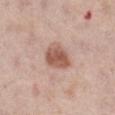No biopsy was performed on this lesion — it was imaged during a full skin examination and was not determined to be concerning. A lesion tile, about 15 mm wide, cut from a 3D total-body photograph. The lesion-visualizer software estimated an area of roughly 9 mm² and an eccentricity of roughly 0.55. The software also gave an average lesion color of about L≈56 a*≈21 b*≈27 (CIELAB), a lesion–skin lightness drop of about 13, and a normalized lesion–skin contrast near 8.5. It also reported an automated nevus-likeness rating near 95 out of 100 and a lesion-detection confidence of about 100/100. The patient is a female in their mid- to late 60s. Imaged with white-light lighting. On the leg. Approximately 3.5 mm at its widest.